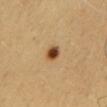Imaged during a routine full-body skin examination; the lesion was not biopsied and no histopathology is available. The tile uses cross-polarized illumination. The patient is a male about 45 years old. Automated image analysis of the tile measured an eccentricity of roughly 0.35 and a shape-asymmetry score of about 0.2 (0 = symmetric). It also reported about 18 CIELAB-L* units darker than the surrounding skin and a normalized border contrast of about 13. It also reported border irregularity of about 1.5 on a 0–10 scale and a color-variation rating of about 5.5/10. A close-up tile cropped from a whole-body skin photograph, about 15 mm across. Longest diameter approximately 2 mm. The lesion is located on the mid back.